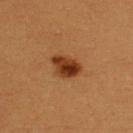Clinical impression: Imaged during a routine full-body skin examination; the lesion was not biopsied and no histopathology is available. Clinical summary: On the upper back. The total-body-photography lesion software estimated a border-irregularity index near 2.5/10 and a color-variation rating of about 5.5/10. Approximately 3.5 mm at its widest. A female subject aged 38 to 42. A roughly 15 mm field-of-view crop from a total-body skin photograph.